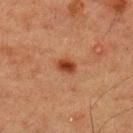Recorded during total-body skin imaging; not selected for excision or biopsy. A male subject aged around 60. A region of skin cropped from a whole-body photographic capture, roughly 15 mm wide. Located on the upper back. This is a cross-polarized tile. An algorithmic analysis of the crop reported a footprint of about 3.5 mm², a shape eccentricity near 0.75, and a shape-asymmetry score of about 0.3 (0 = symmetric). The analysis additionally found a border-irregularity rating of about 2.5/10 and peripheral color asymmetry of about 1.5. The analysis additionally found an automated nevus-likeness rating near 100 out of 100 and lesion-presence confidence of about 100/100. The lesion's longest dimension is about 2.5 mm.